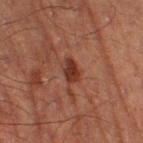{"lesion_size": {"long_diameter_mm_approx": 3.0}, "lighting": "cross-polarized", "site": "right thigh", "patient": {"sex": "male", "age_approx": 70}, "image": {"source": "total-body photography crop", "field_of_view_mm": 15}}